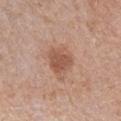Findings:
* biopsy status — total-body-photography surveillance lesion; no biopsy
* body site — the left forearm
* subject — female, aged 58 to 62
* illumination — white-light illumination
* image source — total-body-photography crop, ~15 mm field of view